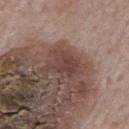Impression:
Captured during whole-body skin photography for melanoma surveillance; the lesion was not biopsied.
Acquisition and patient details:
The subject is a male roughly 75 years of age. On the mid back. Imaged with white-light lighting. The lesion's longest dimension is about 4 mm. A lesion tile, about 15 mm wide, cut from a 3D total-body photograph.